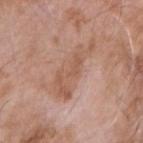This lesion was catalogued during total-body skin photography and was not selected for biopsy. The lesion is on the right upper arm. This image is a 15 mm lesion crop taken from a total-body photograph. The patient is a male in their mid-70s. This is a white-light tile. The lesion's longest dimension is about 6.5 mm.